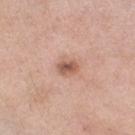Assessment:
This lesion was catalogued during total-body skin photography and was not selected for biopsy.
Clinical summary:
A 15 mm crop from a total-body photograph taken for skin-cancer surveillance. The patient is a female aged 53 to 57. The lesion is on the right thigh. Captured under white-light illumination.